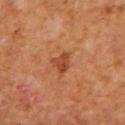The lesion was tiled from a total-body skin photograph and was not biopsied. A male subject, approximately 65 years of age. The lesion is on the mid back. Cropped from a whole-body photographic skin survey; the tile spans about 15 mm. Measured at roughly 2.5 mm in maximum diameter.